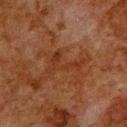biopsy status — catalogued during a skin exam; not biopsied | diameter — ≈5 mm | anatomic site — the back | patient — male, approximately 80 years of age | tile lighting — cross-polarized illumination | image source — total-body-photography crop, ~15 mm field of view.